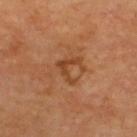workup — no biopsy performed (imaged during a skin exam) | imaging modality — 15 mm crop, total-body photography | lighting — cross-polarized illumination | lesion diameter — ~3.5 mm (longest diameter) | patient — in their mid- to late 60s | anatomic site — the upper back | TBP lesion metrics — an eccentricity of roughly 0.65 and a shape-asymmetry score of about 0.8 (0 = symmetric).